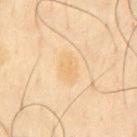biopsy status: total-body-photography surveillance lesion; no biopsy | body site: the front of the torso | patient: male, aged around 65 | lesion size: about 4 mm | acquisition: total-body-photography crop, ~15 mm field of view | TBP lesion metrics: an average lesion color of about L≈72 a*≈14 b*≈40 (CIELAB) and a lesion–skin lightness drop of about 4; border irregularity of about 2 on a 0–10 scale, a within-lesion color-variation index near 2/10, and a peripheral color-asymmetry measure near 1; a lesion-detection confidence of about 100/100 | lighting: cross-polarized illumination.